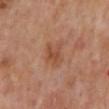<case>
  <biopsy_status>not biopsied; imaged during a skin examination</biopsy_status>
  <patient>
    <sex>male</sex>
    <age_approx>65</age_approx>
  </patient>
  <lesion_size>
    <long_diameter_mm_approx>3.5</long_diameter_mm_approx>
  </lesion_size>
  <site>mid back</site>
  <lighting>cross-polarized</lighting>
  <automated_metrics>
    <border_irregularity_0_10>3.0</border_irregularity_0_10>
    <color_variation_0_10>4.0</color_variation_0_10>
    <peripheral_color_asymmetry>1.5</peripheral_color_asymmetry>
  </automated_metrics>
  <image>
    <source>total-body photography crop</source>
    <field_of_view_mm>15</field_of_view_mm>
  </image>
</case>